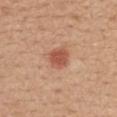Notes:
* notes — total-body-photography surveillance lesion; no biopsy
* image — total-body-photography crop, ~15 mm field of view
* patient — female, about 40 years old
* lighting — white-light illumination
* body site — the back
* size — ≈3 mm
* TBP lesion metrics — a mean CIELAB color near L≈54 a*≈27 b*≈32, roughly 12 lightness units darker than nearby skin, and a normalized border contrast of about 8; a color-variation rating of about 1.5/10 and peripheral color asymmetry of about 0.5